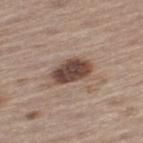Assessment: This lesion was catalogued during total-body skin photography and was not selected for biopsy. Clinical summary: On the left thigh. A 15 mm close-up extracted from a 3D total-body photography capture. A male subject, approximately 70 years of age.